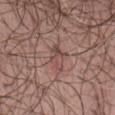Notes:
- notes: no biopsy performed (imaged during a skin exam)
- lesion size: ≈3.5 mm
- automated metrics: a footprint of about 5.5 mm², a shape eccentricity near 0.8, and a shape-asymmetry score of about 0.35 (0 = symmetric); about 7 CIELAB-L* units darker than the surrounding skin and a lesion-to-skin contrast of about 5.5 (normalized; higher = more distinct); internal color variation of about 6 on a 0–10 scale and a peripheral color-asymmetry measure near 2.5; a nevus-likeness score of about 0/100
- site: the abdomen
- subject: male, about 70 years old
- image: ~15 mm tile from a whole-body skin photo